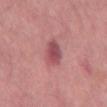{
  "biopsy_status": "not biopsied; imaged during a skin examination",
  "image": {
    "source": "total-body photography crop",
    "field_of_view_mm": 15
  },
  "lighting": "white-light",
  "lesion_size": {
    "long_diameter_mm_approx": 3.5
  },
  "patient": {
    "sex": "male",
    "age_approx": 60
  },
  "site": "mid back"
}